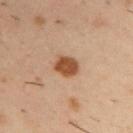<case>
  <biopsy_status>not biopsied; imaged during a skin examination</biopsy_status>
  <image>
    <source>total-body photography crop</source>
    <field_of_view_mm>15</field_of_view_mm>
  </image>
  <patient>
    <sex>male</sex>
    <age_approx>40</age_approx>
  </patient>
  <site>upper back</site>
</case>